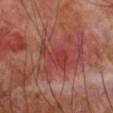Q: Was a biopsy performed?
A: no biopsy performed (imaged during a skin exam)
Q: Lesion location?
A: the right forearm
Q: Automated lesion metrics?
A: an area of roughly 8 mm²
Q: How was this image acquired?
A: ~15 mm crop, total-body skin-cancer survey
Q: Patient demographics?
A: male, aged approximately 70
Q: What is the lesion's diameter?
A: ~4.5 mm (longest diameter)
Q: How was the tile lit?
A: cross-polarized illumination